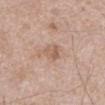<record>
  <biopsy_status>not biopsied; imaged during a skin examination</biopsy_status>
  <patient>
    <sex>male</sex>
    <age_approx>50</age_approx>
  </patient>
  <image>
    <source>total-body photography crop</source>
    <field_of_view_mm>15</field_of_view_mm>
  </image>
  <automated_metrics>
    <area_mm2_approx>4.5</area_mm2_approx>
    <eccentricity>0.6</eccentricity>
    <shape_asymmetry>0.25</shape_asymmetry>
    <cielab_L>59</cielab_L>
    <cielab_a>18</cielab_a>
    <cielab_b>28</cielab_b>
    <vs_skin_darker_L>8.0</vs_skin_darker_L>
    <vs_skin_contrast_norm>5.5</vs_skin_contrast_norm>
    <color_variation_0_10>3.0</color_variation_0_10>
  </automated_metrics>
  <site>chest</site>
</record>